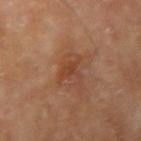Q: Is there a histopathology result?
A: total-body-photography surveillance lesion; no biopsy
Q: How large is the lesion?
A: about 3 mm
Q: What kind of image is this?
A: total-body-photography crop, ~15 mm field of view
Q: Illumination type?
A: cross-polarized
Q: Who is the patient?
A: male, aged approximately 70
Q: What is the anatomic site?
A: the right upper arm